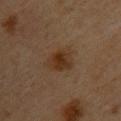Recorded during total-body skin imaging; not selected for excision or biopsy.
The tile uses cross-polarized illumination.
Cropped from a total-body skin-imaging series; the visible field is about 15 mm.
A female subject aged around 45.
From the upper back.